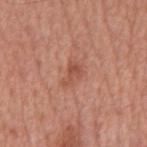The lesion was tiled from a total-body skin photograph and was not biopsied. A 15 mm close-up tile from a total-body photography series done for melanoma screening. A male subject aged 63–67. On the mid back.